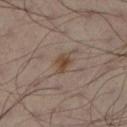{"biopsy_status": "not biopsied; imaged during a skin examination", "image": {"source": "total-body photography crop", "field_of_view_mm": 15}, "patient": {"sex": "male", "age_approx": 50}, "site": "left leg", "lesion_size": {"long_diameter_mm_approx": 2.5}}